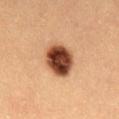follow-up = imaged on a skin check; not biopsied
acquisition = total-body-photography crop, ~15 mm field of view
lesion size = ≈4 mm
patient = male, aged 18–22
location = the lower back